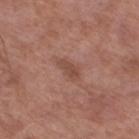Recorded during total-body skin imaging; not selected for excision or biopsy.
Cropped from a whole-body photographic skin survey; the tile spans about 15 mm.
Captured under white-light illumination.
Approximately 2.5 mm at its widest.
The patient is a male aged 53 to 57.
From the leg.
Automated tile analysis of the lesion measured a border-irregularity rating of about 3/10, internal color variation of about 1 on a 0–10 scale, and a peripheral color-asymmetry measure near 0.